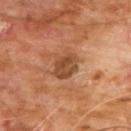follow-up: total-body-photography surveillance lesion; no biopsy
image: ~15 mm tile from a whole-body skin photo
illumination: cross-polarized
subject: male, in their 60s
diameter: ~3.5 mm (longest diameter)
site: the chest